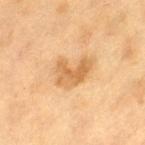Clinical impression:
The lesion was tiled from a total-body skin photograph and was not biopsied.
Acquisition and patient details:
On the leg. A female patient, aged around 40. The recorded lesion diameter is about 4 mm. A close-up tile cropped from a whole-body skin photograph, about 15 mm across. Captured under cross-polarized illumination. The lesion-visualizer software estimated an automated nevus-likeness rating near 15 out of 100.